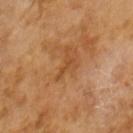<record>
<biopsy_status>not biopsied; imaged during a skin examination</biopsy_status>
<automated_metrics>
  <cielab_L>46</cielab_L>
  <cielab_a>23</cielab_a>
  <cielab_b>39</cielab_b>
  <vs_skin_contrast_norm>5.5</vs_skin_contrast_norm>
  <color_variation_0_10>0.0</color_variation_0_10>
  <peripheral_color_asymmetry>0.0</peripheral_color_asymmetry>
</automated_metrics>
<lighting>cross-polarized</lighting>
<image>
  <source>total-body photography crop</source>
  <field_of_view_mm>15</field_of_view_mm>
</image>
<lesion_size>
  <long_diameter_mm_approx>3.0</long_diameter_mm_approx>
</lesion_size>
<patient>
  <sex>male</sex>
  <age_approx>65</age_approx>
</patient>
</record>